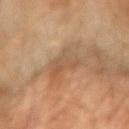Imaged during a routine full-body skin examination; the lesion was not biopsied and no histopathology is available. A roughly 15 mm field-of-view crop from a total-body skin photograph. Imaged with cross-polarized lighting. The lesion is on the right forearm. Approximately 4.5 mm at its widest. A male subject, approximately 60 years of age. The lesion-visualizer software estimated an eccentricity of roughly 0.65 and a symmetry-axis asymmetry near 0.5. The software also gave a lesion–skin lightness drop of about 6.